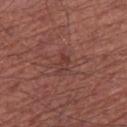Captured during whole-body skin photography for melanoma surveillance; the lesion was not biopsied. A male patient, approximately 65 years of age. This image is a 15 mm lesion crop taken from a total-body photograph. On the leg.